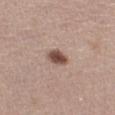imaging modality: 15 mm crop, total-body photography; subject: female, aged approximately 50; tile lighting: white-light illumination; anatomic site: the leg; size: about 2.5 mm.